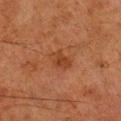No biopsy was performed on this lesion — it was imaged during a full skin examination and was not determined to be concerning. A male patient aged approximately 80. Cropped from a total-body skin-imaging series; the visible field is about 15 mm. Captured under cross-polarized illumination. From the right lower leg. The recorded lesion diameter is about 3 mm. Automated tile analysis of the lesion measured an automated nevus-likeness rating near 5 out of 100 and lesion-presence confidence of about 100/100.